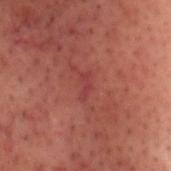<lesion>
  <biopsy_status>not biopsied; imaged during a skin examination</biopsy_status>
</lesion>